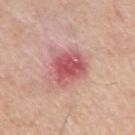{
  "biopsy_status": "not biopsied; imaged during a skin examination",
  "image": {
    "source": "total-body photography crop",
    "field_of_view_mm": 15
  },
  "site": "left upper arm",
  "patient": {
    "sex": "male",
    "age_approx": 55
  }
}